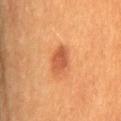Q: Was this lesion biopsied?
A: total-body-photography surveillance lesion; no biopsy
Q: Automated lesion metrics?
A: a shape eccentricity near 0.85; an average lesion color of about L≈52 a*≈29 b*≈38 (CIELAB), roughly 11 lightness units darker than nearby skin, and a normalized lesion–skin contrast near 7.5; a border-irregularity index near 2/10, a within-lesion color-variation index near 3/10, and peripheral color asymmetry of about 1
Q: Patient demographics?
A: male, aged 58 to 62
Q: Lesion size?
A: ≈3.5 mm
Q: How was the tile lit?
A: cross-polarized illumination
Q: What kind of image is this?
A: ~15 mm crop, total-body skin-cancer survey
Q: What is the anatomic site?
A: the lower back